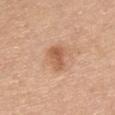| key | value |
|---|---|
| biopsy status | imaged on a skin check; not biopsied |
| site | the chest |
| patient | female, aged approximately 40 |
| image source | 15 mm crop, total-body photography |